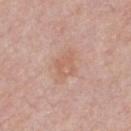<tbp_lesion>
  <site>chest</site>
  <lesion_size>
    <long_diameter_mm_approx>3.0</long_diameter_mm_approx>
  </lesion_size>
  <image>
    <source>total-body photography crop</source>
    <field_of_view_mm>15</field_of_view_mm>
  </image>
  <lighting>white-light</lighting>
  <patient>
    <sex>male</sex>
    <age_approx>60</age_approx>
  </patient>
</tbp_lesion>